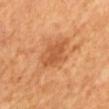This lesion was catalogued during total-body skin photography and was not selected for biopsy. The subject is a male about 65 years old. On the mid back. Captured under cross-polarized illumination. The total-body-photography lesion software estimated an eccentricity of roughly 0.8 and a symmetry-axis asymmetry near 0.25. The software also gave an average lesion color of about L≈56 a*≈28 b*≈41 (CIELAB), a lesion–skin lightness drop of about 10, and a normalized lesion–skin contrast near 6.5. The software also gave a classifier nevus-likeness of about 65/100 and lesion-presence confidence of about 100/100. A 15 mm close-up tile from a total-body photography series done for melanoma screening.